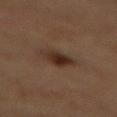Recorded during total-body skin imaging; not selected for excision or biopsy. The recorded lesion diameter is about 3.5 mm. The tile uses cross-polarized illumination. The total-body-photography lesion software estimated an area of roughly 6.5 mm², an outline eccentricity of about 0.8 (0 = round, 1 = elongated), and a shape-asymmetry score of about 0.2 (0 = symmetric). The software also gave an average lesion color of about L≈24 a*≈15 b*≈21 (CIELAB) and a lesion–skin lightness drop of about 9. And it measured a border-irregularity index near 2.5/10, a color-variation rating of about 4/10, and a peripheral color-asymmetry measure near 1. From the mid back. A 15 mm close-up extracted from a 3D total-body photography capture. A male subject aged around 85 or older.